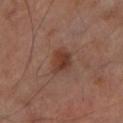  biopsy_status: not biopsied; imaged during a skin examination
  lighting: cross-polarized
  lesion_size:
    long_diameter_mm_approx: 3.5
  site: left lower leg
  patient:
    sex: male
    age_approx: 70
  image:
    source: total-body photography crop
    field_of_view_mm: 15